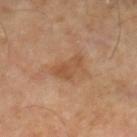Captured during whole-body skin photography for melanoma surveillance; the lesion was not biopsied.